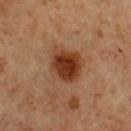workup = total-body-photography surveillance lesion; no biopsy
illumination = cross-polarized
location = the chest
image source = ~15 mm tile from a whole-body skin photo
patient = male, aged 73 to 77
lesion diameter = ≈4 mm